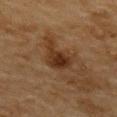Impression:
Imaged during a routine full-body skin examination; the lesion was not biopsied and no histopathology is available.
Background:
Automated tile analysis of the lesion measured a lesion area of about 7.5 mm², an eccentricity of roughly 0.9, and a symmetry-axis asymmetry near 0.45. It also reported a classifier nevus-likeness of about 65/100. The recorded lesion diameter is about 5 mm. Cropped from a total-body skin-imaging series; the visible field is about 15 mm. The patient is a male aged 83 or older. The lesion is on the mid back.